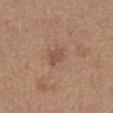The lesion was photographed on a routine skin check and not biopsied; there is no pathology result. Automated tile analysis of the lesion measured a border-irregularity index near 2.5/10, a within-lesion color-variation index near 1.5/10, and peripheral color asymmetry of about 0.5. Located on the mid back. This image is a 15 mm lesion crop taken from a total-body photograph. The lesion's longest dimension is about 2.5 mm. A male patient, about 65 years old.